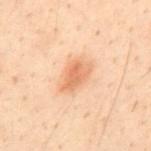notes = total-body-photography surveillance lesion; no biopsy | lesion size = ~4 mm (longest diameter) | site = the mid back | illumination = cross-polarized illumination | imaging modality = 15 mm crop, total-body photography | subject = male, in their 30s.